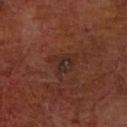Notes:
- workup · no biopsy performed (imaged during a skin exam)
- acquisition · total-body-photography crop, ~15 mm field of view
- patient · male, aged around 65
- location · the left forearm
- image-analysis metrics · an outline eccentricity of about 0.55 (0 = round, 1 = elongated) and a symmetry-axis asymmetry near 0.5; a classifier nevus-likeness of about 5/100
- diameter · ~3.5 mm (longest diameter)
- lighting · cross-polarized illumination